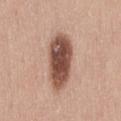The lesion was photographed on a routine skin check and not biopsied; there is no pathology result. A region of skin cropped from a whole-body photographic capture, roughly 15 mm wide. A female patient about 45 years old. Located on the lower back.